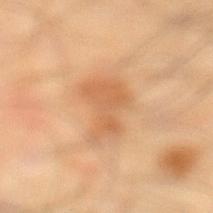<tbp_lesion>
  <biopsy_status>not biopsied; imaged during a skin examination</biopsy_status>
  <image>
    <source>total-body photography crop</source>
    <field_of_view_mm>15</field_of_view_mm>
  </image>
  <site>left lower leg</site>
  <automated_metrics>
    <area_mm2_approx>13.0</area_mm2_approx>
    <eccentricity>0.75</eccentricity>
    <shape_asymmetry>0.45</shape_asymmetry>
    <cielab_L>61</cielab_L>
    <cielab_a>23</cielab_a>
    <cielab_b>39</cielab_b>
    <vs_skin_darker_L>9.0</vs_skin_darker_L>
    <vs_skin_contrast_norm>6.0</vs_skin_contrast_norm>
    <border_irregularity_0_10>5.0</border_irregularity_0_10>
    <color_variation_0_10>2.5</color_variation_0_10>
    <peripheral_color_asymmetry>1.0</peripheral_color_asymmetry>
    <nevus_likeness_0_100>5</nevus_likeness_0_100>
    <lesion_detection_confidence_0_100>100</lesion_detection_confidence_0_100>
  </automated_metrics>
  <lighting>cross-polarized</lighting>
  <lesion_size>
    <long_diameter_mm_approx>5.0</long_diameter_mm_approx>
  </lesion_size>
  <patient>
    <sex>male</sex>
    <age_approx>40</age_approx>
  </patient>
</tbp_lesion>